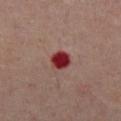Assessment:
Part of a total-body skin-imaging series; this lesion was reviewed on a skin check and was not flagged for biopsy.
Acquisition and patient details:
About 2.5 mm across. A male subject aged 63 to 67. Automated tile analysis of the lesion measured an automated nevus-likeness rating near 0 out of 100. The tile uses cross-polarized illumination. The lesion is on the abdomen. A 15 mm close-up extracted from a 3D total-body photography capture.